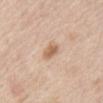biopsy status: total-body-photography surveillance lesion; no biopsy
imaging modality: total-body-photography crop, ~15 mm field of view
anatomic site: the abdomen
subject: female, in their mid- to late 60s
lighting: white-light